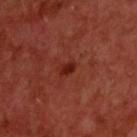No biopsy was performed on this lesion — it was imaged during a full skin examination and was not determined to be concerning.
The subject is a male aged 58–62.
From the upper back.
Longest diameter approximately 2 mm.
A region of skin cropped from a whole-body photographic capture, roughly 15 mm wide.